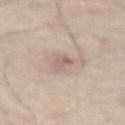No biopsy was performed on this lesion — it was imaged during a full skin examination and was not determined to be concerning. Measured at roughly 2.5 mm in maximum diameter. From the abdomen. A male patient, aged around 80. An algorithmic analysis of the crop reported a lesion color around L≈61 a*≈16 b*≈22 in CIELAB, about 9 CIELAB-L* units darker than the surrounding skin, and a normalized border contrast of about 6. And it measured a border-irregularity rating of about 3/10, a color-variation rating of about 3/10, and radial color variation of about 1. It also reported a nevus-likeness score of about 0/100. A close-up tile cropped from a whole-body skin photograph, about 15 mm across.